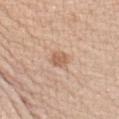Notes:
- notes — total-body-photography surveillance lesion; no biopsy
- image source — 15 mm crop, total-body photography
- anatomic site — the left forearm
- tile lighting — white-light
- patient — female, in their mid- to late 40s
- image-analysis metrics — a nevus-likeness score of about 30/100 and a detector confidence of about 100 out of 100 that the crop contains a lesion